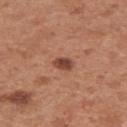| feature | finding |
|---|---|
| follow-up | catalogued during a skin exam; not biopsied |
| subject | female, aged 28–32 |
| image source | ~15 mm tile from a whole-body skin photo |
| anatomic site | the arm |